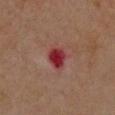Assessment:
The lesion was tiled from a total-body skin photograph and was not biopsied.
Clinical summary:
The lesion is on the chest. Longest diameter approximately 3 mm. A close-up tile cropped from a whole-body skin photograph, about 15 mm across. Captured under cross-polarized illumination. A female subject, aged 68–72.